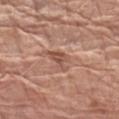Assessment:
Part of a total-body skin-imaging series; this lesion was reviewed on a skin check and was not flagged for biopsy.
Background:
Automated image analysis of the tile measured an average lesion color of about L≈52 a*≈22 b*≈28 (CIELAB), about 10 CIELAB-L* units darker than the surrounding skin, and a normalized border contrast of about 7. The software also gave a within-lesion color-variation index near 1.5/10 and a peripheral color-asymmetry measure near 0.5. About 3 mm across. Cropped from a whole-body photographic skin survey; the tile spans about 15 mm. A male subject, in their 70s. The tile uses white-light illumination. The lesion is on the left upper arm.